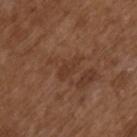This lesion was catalogued during total-body skin photography and was not selected for biopsy. On the arm. The patient is a male approximately 75 years of age. A region of skin cropped from a whole-body photographic capture, roughly 15 mm wide. Longest diameter approximately 3 mm.